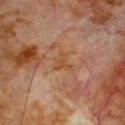A region of skin cropped from a whole-body photographic capture, roughly 15 mm wide. The lesion is located on the chest. The patient is a male aged 78 to 82.